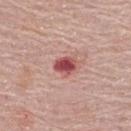No biopsy was performed on this lesion — it was imaged during a full skin examination and was not determined to be concerning.
A female patient, in their mid- to late 60s.
Approximately 2.5 mm at its widest.
Automated image analysis of the tile measured a footprint of about 5 mm², an eccentricity of roughly 0.6, and a symmetry-axis asymmetry near 0.2. And it measured about 16 CIELAB-L* units darker than the surrounding skin and a lesion-to-skin contrast of about 10.5 (normalized; higher = more distinct). The software also gave lesion-presence confidence of about 100/100.
Cropped from a whole-body photographic skin survey; the tile spans about 15 mm.
The tile uses white-light illumination.
The lesion is located on the upper back.